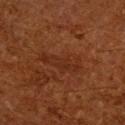Imaged during a routine full-body skin examination; the lesion was not biopsied and no histopathology is available.